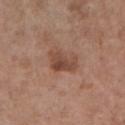The lesion was tiled from a total-body skin photograph and was not biopsied. A female patient, roughly 75 years of age. Imaged with white-light lighting. The lesion is located on the chest. The lesion-visualizer software estimated a lesion area of about 6.5 mm². A close-up tile cropped from a whole-body skin photograph, about 15 mm across.